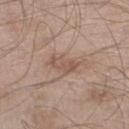biopsy_status: not biopsied; imaged during a skin examination
site: left lower leg
patient:
  sex: male
  age_approx: 55
automated_metrics:
  area_mm2_approx: 5.5
  shape_asymmetry: 0.4
  cielab_L: 54
  cielab_a: 17
  cielab_b: 26
  vs_skin_darker_L: 8.0
  vs_skin_contrast_norm: 6.0
lighting: white-light
image:
  source: total-body photography crop
  field_of_view_mm: 15